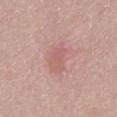| feature | finding |
|---|---|
| illumination | white-light |
| location | the abdomen |
| patient | male, aged around 55 |
| automated lesion analysis | an area of roughly 8 mm², an outline eccentricity of about 0.9 (0 = round, 1 = elongated), and a symmetry-axis asymmetry near 0.25; a lesion color around L≈60 a*≈24 b*≈23 in CIELAB and a normalized lesion–skin contrast near 4.5; lesion-presence confidence of about 100/100 |
| image source | total-body-photography crop, ~15 mm field of view |